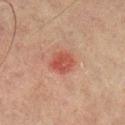notes=no biopsy performed (imaged during a skin exam)
lesion diameter=≈3.5 mm
imaging modality=total-body-photography crop, ~15 mm field of view
automated metrics=an area of roughly 6.5 mm²; an automated nevus-likeness rating near 85 out of 100 and a lesion-detection confidence of about 100/100
anatomic site=the right lower leg
patient=male, aged 73–77
lighting=cross-polarized